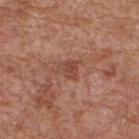biopsy status = total-body-photography surveillance lesion; no biopsy | image = ~15 mm tile from a whole-body skin photo | patient = male, aged 63–67 | body site = the back | illumination = white-light illumination | diameter = ≈2.5 mm.